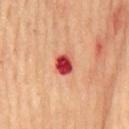The lesion is located on the chest.
A lesion tile, about 15 mm wide, cut from a 3D total-body photograph.
A male subject, aged around 65.
Captured under cross-polarized illumination.